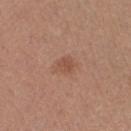No biopsy was performed on this lesion — it was imaged during a full skin examination and was not determined to be concerning. A female patient, aged 38–42. The recorded lesion diameter is about 3 mm. From the right lower leg. Cropped from a whole-body photographic skin survey; the tile spans about 15 mm. Imaged with white-light lighting.